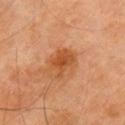This lesion was catalogued during total-body skin photography and was not selected for biopsy.
The recorded lesion diameter is about 3.5 mm.
A 15 mm crop from a total-body photograph taken for skin-cancer surveillance.
The lesion is located on the right upper arm.
A male patient aged approximately 65.